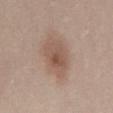workup=total-body-photography surveillance lesion; no biopsy
location=the abdomen
image source=~15 mm tile from a whole-body skin photo
patient=female, aged approximately 25